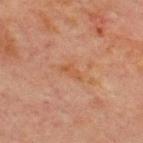Located on the chest.
A male patient, aged approximately 70.
Longest diameter approximately 3 mm.
This image is a 15 mm lesion crop taken from a total-body photograph.